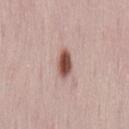workup=catalogued during a skin exam; not biopsied | acquisition=~15 mm crop, total-body skin-cancer survey | anatomic site=the back | subject=female, roughly 30 years of age.